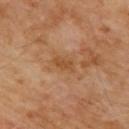  biopsy_status: not biopsied; imaged during a skin examination
  automated_metrics:
    eccentricity: 0.8
    shape_asymmetry: 0.25
  patient:
    sex: male
    age_approx: 70
  site: upper back
  image:
    source: total-body photography crop
    field_of_view_mm: 15